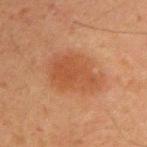notes: imaged on a skin check; not biopsied
patient: male, in their mid-40s
site: the right upper arm
image source: ~15 mm crop, total-body skin-cancer survey